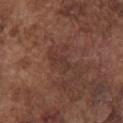workup — total-body-photography surveillance lesion; no biopsy
subject — male, aged around 75
imaging modality — ~15 mm crop, total-body skin-cancer survey
site — the chest
automated lesion analysis — a footprint of about 2.5 mm², an eccentricity of roughly 0.9, and a shape-asymmetry score of about 0.5 (0 = symmetric); a mean CIELAB color near L≈34 a*≈20 b*≈22
illumination — white-light illumination